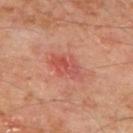{"biopsy_status": "not biopsied; imaged during a skin examination", "automated_metrics": {"area_mm2_approx": 7.5, "eccentricity": 0.85, "shape_asymmetry": 0.35, "cielab_L": 52, "cielab_a": 31, "cielab_b": 30, "border_irregularity_0_10": 4.5, "peripheral_color_asymmetry": 2.0}, "image": {"source": "total-body photography crop", "field_of_view_mm": 15}, "patient": {"sex": "male", "age_approx": 65}, "site": "mid back", "lesion_size": {"long_diameter_mm_approx": 4.5}, "lighting": "cross-polarized"}